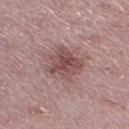notes: imaged on a skin check; not biopsied | illumination: white-light illumination | patient: female, in their mid-40s | body site: the left lower leg | image source: ~15 mm tile from a whole-body skin photo | automated metrics: an area of roughly 11 mm², an outline eccentricity of about 0.45 (0 = round, 1 = elongated), and a shape-asymmetry score of about 0.25 (0 = symmetric); a lesion–skin lightness drop of about 11 and a normalized border contrast of about 8; a border-irregularity index near 3.5/10, a within-lesion color-variation index near 4/10, and radial color variation of about 1.5; a detector confidence of about 100 out of 100 that the crop contains a lesion | lesion size: about 4 mm.